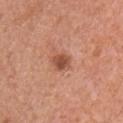No biopsy was performed on this lesion — it was imaged during a full skin examination and was not determined to be concerning. The subject is a male about 55 years old. Located on the chest. Captured under white-light illumination. A 15 mm close-up tile from a total-body photography series done for melanoma screening.